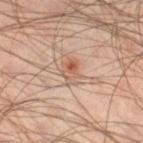Captured during whole-body skin photography for melanoma surveillance; the lesion was not biopsied. A 15 mm crop from a total-body photograph taken for skin-cancer surveillance. The tile uses cross-polarized illumination. A male patient in their mid-40s. On the right thigh. The lesion's longest dimension is about 3 mm. Automated tile analysis of the lesion measured a footprint of about 5 mm² and an outline eccentricity of about 0.75 (0 = round, 1 = elongated). The analysis additionally found an automated nevus-likeness rating near 20 out of 100 and a detector confidence of about 100 out of 100 that the crop contains a lesion.